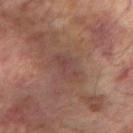{"biopsy_status": "not biopsied; imaged during a skin examination", "lighting": "cross-polarized", "patient": {"sex": "male", "age_approx": 65}, "lesion_size": {"long_diameter_mm_approx": 4.5}, "site": "right forearm", "automated_metrics": {"cielab_L": 42, "cielab_a": 19, "cielab_b": 21, "vs_skin_darker_L": 6.0, "vs_skin_contrast_norm": 5.5}, "image": {"source": "total-body photography crop", "field_of_view_mm": 15}}